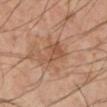  biopsy_status: not biopsied; imaged during a skin examination
  site: left lower leg
  patient:
    sex: male
    age_approx: 50
  lesion_size:
    long_diameter_mm_approx: 4.5
  image:
    source: total-body photography crop
    field_of_view_mm: 15
  lighting: cross-polarized